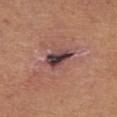The lesion was photographed on a routine skin check and not biopsied; there is no pathology result. A lesion tile, about 15 mm wide, cut from a 3D total-body photograph. This is a white-light tile. A female subject roughly 50 years of age. Automated image analysis of the tile measured a classifier nevus-likeness of about 0/100. Located on the chest. Measured at roughly 3.5 mm in maximum diameter.